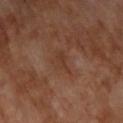| field | value |
|---|---|
| follow-up | imaged on a skin check; not biopsied |
| image source | 15 mm crop, total-body photography |
| tile lighting | cross-polarized illumination |
| body site | the back |
| automated metrics | an area of roughly 4.5 mm², an outline eccentricity of about 0.75 (0 = round, 1 = elongated), and two-axis asymmetry of about 0.45; a mean CIELAB color near L≈36 a*≈20 b*≈27; a nevus-likeness score of about 0/100 and lesion-presence confidence of about 100/100 |
| subject | male, aged around 70 |
| lesion size | ~3 mm (longest diameter) |